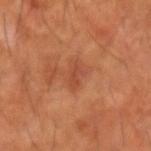notes=catalogued during a skin exam; not biopsied | acquisition=15 mm crop, total-body photography | body site=the arm | image-analysis metrics=an area of roughly 3.5 mm² and a symmetry-axis asymmetry near 0.35; an average lesion color of about L≈44 a*≈28 b*≈33 (CIELAB) and about 7 CIELAB-L* units darker than the surrounding skin; a nevus-likeness score of about 0/100 and a detector confidence of about 100 out of 100 that the crop contains a lesion | subject=male, roughly 60 years of age.